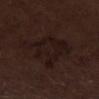biopsy status: total-body-photography surveillance lesion; no biopsy | automated lesion analysis: a lesion color around L≈14 a*≈13 b*≈14 in CIELAB and a lesion–skin lightness drop of about 4 | diameter: about 6 mm | imaging modality: ~15 mm crop, total-body skin-cancer survey | site: the left lower leg | subject: male, aged around 70 | lighting: white-light.